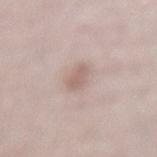Part of a total-body skin-imaging series; this lesion was reviewed on a skin check and was not flagged for biopsy. A lesion tile, about 15 mm wide, cut from a 3D total-body photograph. The lesion is located on the mid back. About 2.5 mm across. A male patient, roughly 70 years of age.